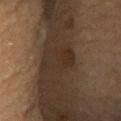Imaged during a routine full-body skin examination; the lesion was not biopsied and no histopathology is available.
On the chest.
A lesion tile, about 15 mm wide, cut from a 3D total-body photograph.
A female patient, aged 58 to 62.
The recorded lesion diameter is about 4.5 mm.